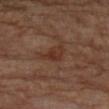workup: imaged on a skin check; not biopsied | patient: male, roughly 85 years of age or older | anatomic site: the left forearm | acquisition: 15 mm crop, total-body photography.